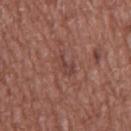biopsy status: catalogued during a skin exam; not biopsied | subject: male, in their mid- to late 70s | image: ~15 mm tile from a whole-body skin photo | location: the chest | lesion diameter: about 3 mm.